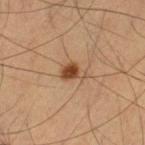Assessment:
The lesion was tiled from a total-body skin photograph and was not biopsied.
Context:
Captured under cross-polarized illumination. On the right thigh. A 15 mm crop from a total-body photograph taken for skin-cancer surveillance. A male subject, aged 58–62. Automated image analysis of the tile measured roughly 12 lightness units darker than nearby skin and a normalized lesion–skin contrast near 9.5. The software also gave an automated nevus-likeness rating near 95 out of 100 and a lesion-detection confidence of about 100/100. The lesion's longest dimension is about 3 mm.